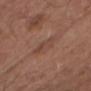biopsy_status: not biopsied; imaged during a skin examination
lighting: white-light
site: head or neck
patient:
  sex: male
  age_approx: 55
image:
  source: total-body photography crop
  field_of_view_mm: 15